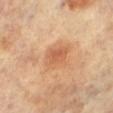<tbp_lesion>
  <biopsy_status>not biopsied; imaged during a skin examination</biopsy_status>
  <patient>
    <sex>female</sex>
    <age_approx>65</age_approx>
  </patient>
  <site>right leg</site>
  <image>
    <source>total-body photography crop</source>
    <field_of_view_mm>15</field_of_view_mm>
  </image>
  <lesion_size>
    <long_diameter_mm_approx>3.0</long_diameter_mm_approx>
  </lesion_size>
  <lighting>cross-polarized</lighting>
  <automated_metrics>
    <cielab_L>58</cielab_L>
    <cielab_a>24</cielab_a>
    <cielab_b>37</cielab_b>
    <vs_skin_contrast_norm>6.0</vs_skin_contrast_norm>
    <border_irregularity_0_10>2.0</border_irregularity_0_10>
    <peripheral_color_asymmetry>1.0</peripheral_color_asymmetry>
  </automated_metrics>
</tbp_lesion>